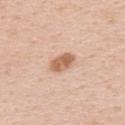Located on the upper back.
Captured under white-light illumination.
A male patient aged 48 to 52.
About 3 mm across.
Cropped from a whole-body photographic skin survey; the tile spans about 15 mm.